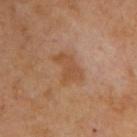This lesion was catalogued during total-body skin photography and was not selected for biopsy.
Longest diameter approximately 3.5 mm.
A subject approximately 65 years of age.
A roughly 15 mm field-of-view crop from a total-body skin photograph.
The lesion is on the upper back.